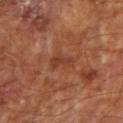Findings:
– workup: imaged on a skin check; not biopsied
– imaging modality: total-body-photography crop, ~15 mm field of view
– subject: male, in their mid-60s
– illumination: cross-polarized
– body site: the right upper arm
– lesion diameter: about 3 mm